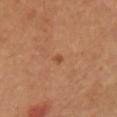Clinical impression: Recorded during total-body skin imaging; not selected for excision or biopsy. Clinical summary: A female subject aged 48 to 52. A 15 mm crop from a total-body photograph taken for skin-cancer surveillance. On the front of the torso.